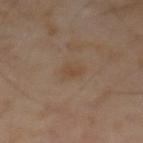image source: 15 mm crop, total-body photography | subject: male, roughly 65 years of age | lesion size: about 3 mm | image-analysis metrics: a lesion area of about 5 mm², an outline eccentricity of about 0.5 (0 = round, 1 = elongated), and a symmetry-axis asymmetry near 0.25; roughly 5 lightness units darker than nearby skin and a normalized lesion–skin contrast near 5; border irregularity of about 2.5 on a 0–10 scale, internal color variation of about 2 on a 0–10 scale, and radial color variation of about 0.5 | lighting: cross-polarized illumination.